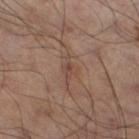{"biopsy_status": "not biopsied; imaged during a skin examination", "patient": {"sex": "male", "age_approx": 50}, "lesion_size": {"long_diameter_mm_approx": 3.0}, "image": {"source": "total-body photography crop", "field_of_view_mm": 15}, "automated_metrics": {"area_mm2_approx": 3.0, "shape_asymmetry": 0.4, "cielab_L": 44, "cielab_a": 18, "cielab_b": 24, "vs_skin_darker_L": 6.0, "vs_skin_contrast_norm": 5.0, "border_irregularity_0_10": 4.5, "color_variation_0_10": 3.0, "peripheral_color_asymmetry": 1.5, "nevus_likeness_0_100": 0}, "lighting": "cross-polarized", "site": "leg"}